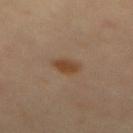Impression: Imaged during a routine full-body skin examination; the lesion was not biopsied and no histopathology is available. Clinical summary: From the mid back. A male subject aged around 40. Captured under cross-polarized illumination. A 15 mm close-up extracted from a 3D total-body photography capture.